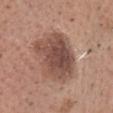notes=no biopsy performed (imaged during a skin exam) | location=the head or neck | size=~6.5 mm (longest diameter) | illumination=white-light illumination | automated metrics=an area of roughly 24 mm², an eccentricity of roughly 0.65, and a symmetry-axis asymmetry near 0.2; a mean CIELAB color near L≈48 a*≈20 b*≈25 and a normalized border contrast of about 9 | subject=male, in their 50s | imaging modality=~15 mm crop, total-body skin-cancer survey.